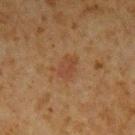A lesion tile, about 15 mm wide, cut from a 3D total-body photograph.
The patient is a male aged 58–62.
Longest diameter approximately 3 mm.
Located on the left upper arm.
This is a cross-polarized tile.